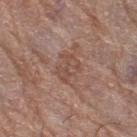This lesion was catalogued during total-body skin photography and was not selected for biopsy.
A lesion tile, about 15 mm wide, cut from a 3D total-body photograph.
Approximately 3 mm at its widest.
From the right thigh.
The subject is a female aged 78 to 82.